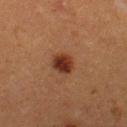Q: Was a biopsy performed?
A: total-body-photography surveillance lesion; no biopsy
Q: What is the imaging modality?
A: 15 mm crop, total-body photography
Q: Lesion location?
A: the left thigh
Q: Patient demographics?
A: female, in their 40s
Q: What lighting was used for the tile?
A: cross-polarized illumination